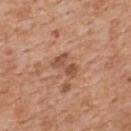Assessment: Recorded during total-body skin imaging; not selected for excision or biopsy. Background: The lesion is on the upper back. A male patient, in their 60s. Imaged with white-light lighting. A 15 mm close-up tile from a total-body photography series done for melanoma screening. Approximately 3.5 mm at its widest.